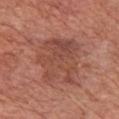Impression: Part of a total-body skin-imaging series; this lesion was reviewed on a skin check and was not flagged for biopsy. Background: A male patient, about 75 years old. Approximately 6 mm at its widest. An algorithmic analysis of the crop reported a lesion area of about 25 mm², an eccentricity of roughly 0.4, and a symmetry-axis asymmetry near 0.25. It also reported a lesion–skin lightness drop of about 7 and a normalized border contrast of about 5.5. Located on the chest. Cropped from a total-body skin-imaging series; the visible field is about 15 mm. Imaged with white-light lighting.